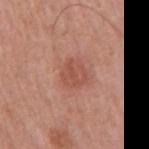{"biopsy_status": "not biopsied; imaged during a skin examination", "site": "right upper arm", "image": {"source": "total-body photography crop", "field_of_view_mm": 15}, "patient": {"sex": "male", "age_approx": 55}, "lighting": "white-light"}